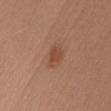biopsy status = no biopsy performed (imaged during a skin exam) | subject = female, aged 43–47 | automated metrics = a lesion area of about 5 mm²; a normalized lesion–skin contrast near 6; a nevus-likeness score of about 75/100 and lesion-presence confidence of about 100/100 | location = the chest | image = total-body-photography crop, ~15 mm field of view | lesion diameter = about 3 mm | illumination = white-light illumination.